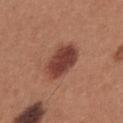This lesion was catalogued during total-body skin photography and was not selected for biopsy. The subject is a male aged around 35. Approximately 5 mm at its widest. An algorithmic analysis of the crop reported a mean CIELAB color near L≈42 a*≈25 b*≈26, about 14 CIELAB-L* units darker than the surrounding skin, and a normalized border contrast of about 10.5. And it measured a border-irregularity index near 1.5/10, internal color variation of about 4 on a 0–10 scale, and peripheral color asymmetry of about 1.5. It also reported lesion-presence confidence of about 100/100. Captured under white-light illumination. On the front of the torso. A 15 mm close-up extracted from a 3D total-body photography capture.